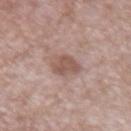{"image": {"source": "total-body photography crop", "field_of_view_mm": 15}, "patient": {"sex": "male", "age_approx": 55}, "lighting": "white-light", "lesion_size": {"long_diameter_mm_approx": 3.0}, "site": "right upper arm"}